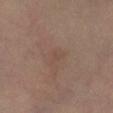The lesion was tiled from a total-body skin photograph and was not biopsied. The lesion is on the left lower leg. An algorithmic analysis of the crop reported a mean CIELAB color near L≈44 a*≈16 b*≈23 and a normalized border contrast of about 3. It also reported a border-irregularity rating of about 3/10 and radial color variation of about 0. The patient is a male roughly 60 years of age. This is a cross-polarized tile. A lesion tile, about 15 mm wide, cut from a 3D total-body photograph.